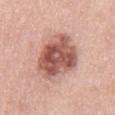Part of a total-body skin-imaging series; this lesion was reviewed on a skin check and was not flagged for biopsy. A lesion tile, about 15 mm wide, cut from a 3D total-body photograph. Imaged with white-light lighting. Located on the chest. A female subject, in their 40s.